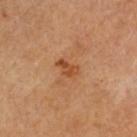<record>
  <biopsy_status>not biopsied; imaged during a skin examination</biopsy_status>
  <patient>
    <sex>female</sex>
    <age_approx>65</age_approx>
  </patient>
  <site>head or neck</site>
  <lesion_size>
    <long_diameter_mm_approx>2.5</long_diameter_mm_approx>
  </lesion_size>
  <automated_metrics>
    <cielab_L>47</cielab_L>
    <cielab_a>25</cielab_a>
    <cielab_b>38</cielab_b>
    <vs_skin_contrast_norm>7.5</vs_skin_contrast_norm>
    <nevus_likeness_0_100>35</nevus_likeness_0_100>
  </automated_metrics>
  <image>
    <source>total-body photography crop</source>
    <field_of_view_mm>15</field_of_view_mm>
  </image>
  <lighting>cross-polarized</lighting>
</record>